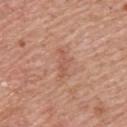{
  "biopsy_status": "not biopsied; imaged during a skin examination",
  "site": "upper back",
  "image": {
    "source": "total-body photography crop",
    "field_of_view_mm": 15
  },
  "lighting": "white-light",
  "lesion_size": {
    "long_diameter_mm_approx": 3.5
  },
  "patient": {
    "sex": "male",
    "age_approx": 60
  }
}